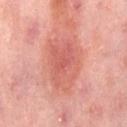Clinical impression:
Imaged during a routine full-body skin examination; the lesion was not biopsied and no histopathology is available.
Clinical summary:
Automated image analysis of the tile measured a mean CIELAB color near L≈58 a*≈30 b*≈28, a lesion–skin lightness drop of about 9, and a lesion-to-skin contrast of about 6 (normalized; higher = more distinct). The software also gave a nevus-likeness score of about 65/100. Cropped from a total-body skin-imaging series; the visible field is about 15 mm. From the abdomen. The lesion's longest dimension is about 6.5 mm. Imaged with cross-polarized lighting. A female patient aged 48–52.